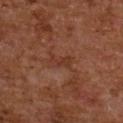Assessment: The lesion was photographed on a routine skin check and not biopsied; there is no pathology result. Acquisition and patient details: A female patient, approximately 60 years of age. The recorded lesion diameter is about 3 mm. A 15 mm close-up extracted from a 3D total-body photography capture. This is a cross-polarized tile. Automated tile analysis of the lesion measured a mean CIELAB color near L≈30 a*≈21 b*≈26, about 5 CIELAB-L* units darker than the surrounding skin, and a lesion-to-skin contrast of about 5 (normalized; higher = more distinct). The analysis additionally found border irregularity of about 4.5 on a 0–10 scale. The lesion is on the back.